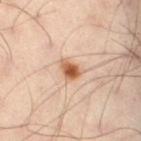No biopsy was performed on this lesion — it was imaged during a full skin examination and was not determined to be concerning. Cropped from a total-body skin-imaging series; the visible field is about 15 mm. Longest diameter approximately 2.5 mm. Captured under cross-polarized illumination. A male subject approximately 50 years of age. The lesion is located on the back.